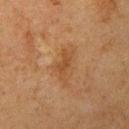* biopsy status · no biopsy performed (imaged during a skin exam)
* diameter · about 3.5 mm
* image-analysis metrics · an outline eccentricity of about 0.9 (0 = round, 1 = elongated) and two-axis asymmetry of about 0.4
* patient · female, about 60 years old
* image source · total-body-photography crop, ~15 mm field of view
* tile lighting · cross-polarized
* site · the right upper arm